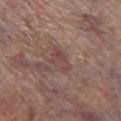Recorded during total-body skin imaging; not selected for excision or biopsy.
Cropped from a whole-body photographic skin survey; the tile spans about 15 mm.
From the right lower leg.
A male patient, about 70 years old.
About 3.5 mm across.